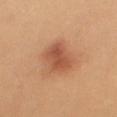workup: catalogued during a skin exam; not biopsied
subject: female, roughly 60 years of age
acquisition: ~15 mm crop, total-body skin-cancer survey
diameter: ~3.5 mm (longest diameter)
illumination: cross-polarized illumination
body site: the left leg